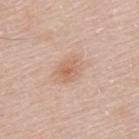Imaged during a routine full-body skin examination; the lesion was not biopsied and no histopathology is available. A lesion tile, about 15 mm wide, cut from a 3D total-body photograph. A male subject roughly 50 years of age. From the mid back. Imaged with white-light lighting. The lesion's longest dimension is about 4.5 mm.